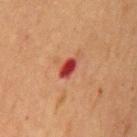Impression:
No biopsy was performed on this lesion — it was imaged during a full skin examination and was not determined to be concerning.
Clinical summary:
A male patient, aged 63–67. Cropped from a whole-body photographic skin survey; the tile spans about 15 mm. On the front of the torso.